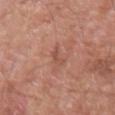A 15 mm close-up tile from a total-body photography series done for melanoma screening. A male subject, in their 80s. On the arm.